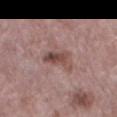Clinical impression: No biopsy was performed on this lesion — it was imaged during a full skin examination and was not determined to be concerning. Background: The total-body-photography lesion software estimated a footprint of about 6.5 mm² and two-axis asymmetry of about 0.4. And it measured border irregularity of about 4.5 on a 0–10 scale, a within-lesion color-variation index near 5.5/10, and peripheral color asymmetry of about 2. The patient is a male aged approximately 70. The lesion is located on the leg. A lesion tile, about 15 mm wide, cut from a 3D total-body photograph. This is a white-light tile. About 4 mm across.